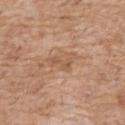notes=imaged on a skin check; not biopsied
image=15 mm crop, total-body photography
lighting=white-light
patient=male, in their 60s
site=the chest